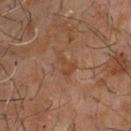Cropped from a total-body skin-imaging series; the visible field is about 15 mm.
The patient is a male approximately 60 years of age.
Located on the front of the torso.
Automated tile analysis of the lesion measured an area of roughly 4 mm² and an eccentricity of roughly 0.85. The software also gave border irregularity of about 6 on a 0–10 scale and radial color variation of about 0.
The tile uses cross-polarized illumination.
Approximately 3 mm at its widest.